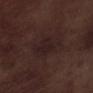The lesion was tiled from a total-body skin photograph and was not biopsied. Cropped from a total-body skin-imaging series; the visible field is about 15 mm. A male subject, aged approximately 70. Located on the leg.